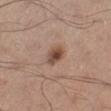Assessment: Imaged during a routine full-body skin examination; the lesion was not biopsied and no histopathology is available. Acquisition and patient details: On the left lower leg. A 15 mm close-up tile from a total-body photography series done for melanoma screening. A male patient, aged approximately 60. The tile uses white-light illumination.